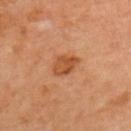{
  "biopsy_status": "not biopsied; imaged during a skin examination",
  "site": "left upper arm",
  "lesion_size": {
    "long_diameter_mm_approx": 3.5
  },
  "image": {
    "source": "total-body photography crop",
    "field_of_view_mm": 15
  },
  "patient": {
    "sex": "female",
    "age_approx": 50
  },
  "lighting": "cross-polarized",
  "automated_metrics": {
    "area_mm2_approx": 5.5,
    "shape_asymmetry": 0.25,
    "border_irregularity_0_10": 2.5,
    "color_variation_0_10": 3.0,
    "peripheral_color_asymmetry": 1.0
  }
}